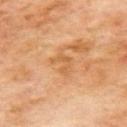Notes:
– workup — imaged on a skin check; not biopsied
– lighting — cross-polarized illumination
– anatomic site — the back
– acquisition — ~15 mm crop, total-body skin-cancer survey
– subject — male, aged around 70
– diameter — about 3 mm